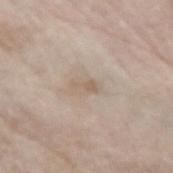The lesion was tiled from a total-body skin photograph and was not biopsied. The subject is a male roughly 80 years of age. A lesion tile, about 15 mm wide, cut from a 3D total-body photograph. Imaged with white-light lighting. An algorithmic analysis of the crop reported a lesion color around L≈60 a*≈13 b*≈26 in CIELAB, a lesion–skin lightness drop of about 8, and a normalized border contrast of about 5.5. The analysis additionally found internal color variation of about 0 on a 0–10 scale. The software also gave a nevus-likeness score of about 0/100 and lesion-presence confidence of about 80/100. Located on the left forearm.